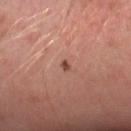{
  "biopsy_status": "not biopsied; imaged during a skin examination",
  "automated_metrics": {
    "color_variation_0_10": 0.0,
    "peripheral_color_asymmetry": 0.0
  },
  "site": "left forearm",
  "image": {
    "source": "total-body photography crop",
    "field_of_view_mm": 15
  },
  "patient": {
    "sex": "male",
    "age_approx": 30
  }
}